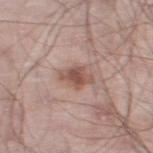Imaged during a routine full-body skin examination; the lesion was not biopsied and no histopathology is available.